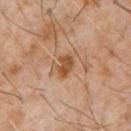| feature | finding |
|---|---|
| follow-up | no biopsy performed (imaged during a skin exam) |
| site | the chest |
| subject | male, aged 58–62 |
| image | ~15 mm tile from a whole-body skin photo |
| illumination | cross-polarized illumination |
| lesion size | about 3 mm |
| automated lesion analysis | a border-irregularity index near 3/10 and radial color variation of about 0.5; a nevus-likeness score of about 55/100 and a detector confidence of about 100 out of 100 that the crop contains a lesion |